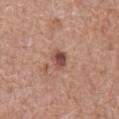Q: Is there a histopathology result?
A: imaged on a skin check; not biopsied
Q: What is the lesion's diameter?
A: ~2.5 mm (longest diameter)
Q: Patient demographics?
A: male, aged 73 to 77
Q: What is the imaging modality?
A: ~15 mm crop, total-body skin-cancer survey
Q: What is the anatomic site?
A: the abdomen
Q: What did automated image analysis measure?
A: a footprint of about 4.5 mm², an eccentricity of roughly 0.55, and a shape-asymmetry score of about 0.25 (0 = symmetric); a mean CIELAB color near L≈49 a*≈22 b*≈26, a lesion–skin lightness drop of about 12, and a lesion-to-skin contrast of about 9 (normalized; higher = more distinct); a border-irregularity rating of about 2/10, a within-lesion color-variation index near 4.5/10, and radial color variation of about 1.5; a lesion-detection confidence of about 100/100
Q: How was the tile lit?
A: white-light